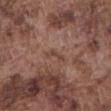follow-up = total-body-photography surveillance lesion; no biopsy | lighting = white-light | patient = male, about 75 years old | acquisition = ~15 mm crop, total-body skin-cancer survey | body site = the abdomen | lesion size = about 2.5 mm.